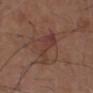Located on the chest. A lesion tile, about 15 mm wide, cut from a 3D total-body photograph. The lesion's longest dimension is about 6 mm. The subject is a male approximately 75 years of age. Imaged with white-light lighting. The lesion-visualizer software estimated a footprint of about 9 mm², an eccentricity of roughly 0.95, and a shape-asymmetry score of about 0.45 (0 = symmetric).